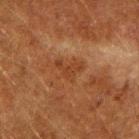Imaged during a routine full-body skin examination; the lesion was not biopsied and no histopathology is available.
A close-up tile cropped from a whole-body skin photograph, about 15 mm across.
An algorithmic analysis of the crop reported a footprint of about 4.5 mm², a shape eccentricity near 0.75, and a symmetry-axis asymmetry near 0.7. The software also gave a border-irregularity rating of about 7/10, a color-variation rating of about 0.5/10, and radial color variation of about 0.5. It also reported a nevus-likeness score of about 0/100 and lesion-presence confidence of about 100/100.
A male subject, approximately 60 years of age.
Captured under cross-polarized illumination.
From the right upper arm.
Approximately 3 mm at its widest.